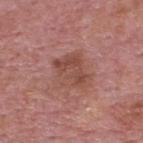Q: Is there a histopathology result?
A: no biopsy performed (imaged during a skin exam)
Q: How was this image acquired?
A: 15 mm crop, total-body photography
Q: Where on the body is the lesion?
A: the upper back
Q: What lighting was used for the tile?
A: white-light
Q: What are the patient's age and sex?
A: male, in their mid- to late 70s
Q: How large is the lesion?
A: ≈4 mm